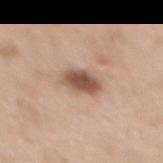notes: no biopsy performed (imaged during a skin exam).